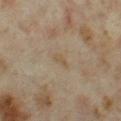Assessment:
Part of a total-body skin-imaging series; this lesion was reviewed on a skin check and was not flagged for biopsy.
Background:
A female subject aged 33 to 37. On the left thigh. The lesion-visualizer software estimated an area of roughly 2.5 mm², a shape eccentricity near 0.9, and two-axis asymmetry of about 0.35. The software also gave an automated nevus-likeness rating near 0 out of 100 and lesion-presence confidence of about 100/100. Approximately 2.5 mm at its widest. A 15 mm close-up tile from a total-body photography series done for melanoma screening. Captured under cross-polarized illumination.